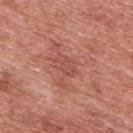follow-up — imaged on a skin check; not biopsied | illumination — white-light | patient — male, approximately 70 years of age | lesion size — ~3 mm (longest diameter) | location — the upper back | automated lesion analysis — a shape eccentricity near 0.8 and two-axis asymmetry of about 0.35 | imaging modality — ~15 mm crop, total-body skin-cancer survey.